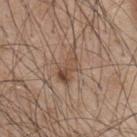Case summary:
- workup · no biopsy performed (imaged during a skin exam)
- diameter · ~3.5 mm (longest diameter)
- acquisition · ~15 mm crop, total-body skin-cancer survey
- site · the upper back
- tile lighting · white-light
- subject · male, aged around 45
- automated metrics · a lesion color around L≈48 a*≈17 b*≈28 in CIELAB, a lesion–skin lightness drop of about 9, and a normalized border contrast of about 7; a border-irregularity index near 4.5/10 and radial color variation of about 3